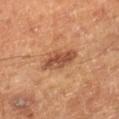notes = imaged on a skin check; not biopsied | subject = male, aged 58 to 62 | diameter = ~4 mm (longest diameter) | site = the leg | automated metrics = a footprint of about 10 mm², an eccentricity of roughly 0.75, and a symmetry-axis asymmetry near 0.2; an automated nevus-likeness rating near 75 out of 100 and a detector confidence of about 100 out of 100 that the crop contains a lesion | acquisition = 15 mm crop, total-body photography.